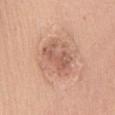Case summary:
– workup · imaged on a skin check; not biopsied
– anatomic site · the chest
– subject · female, about 60 years old
– acquisition · 15 mm crop, total-body photography